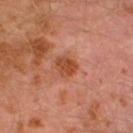Assessment:
Captured during whole-body skin photography for melanoma surveillance; the lesion was not biopsied.
Background:
About 2.5 mm across. From the left lower leg. Imaged with cross-polarized lighting. Cropped from a total-body skin-imaging series; the visible field is about 15 mm. A male patient, in their 30s.